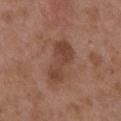Q: Was this lesion biopsied?
A: no biopsy performed (imaged during a skin exam)
Q: Patient demographics?
A: female, roughly 35 years of age
Q: Lesion size?
A: ≈6 mm
Q: How was this image acquired?
A: ~15 mm tile from a whole-body skin photo
Q: What did automated image analysis measure?
A: a footprint of about 11 mm² and a shape eccentricity near 0.9; a mean CIELAB color near L≈43 a*≈21 b*≈28, roughly 9 lightness units darker than nearby skin, and a normalized lesion–skin contrast near 7; a border-irregularity rating of about 4.5/10 and internal color variation of about 3 on a 0–10 scale
Q: What is the anatomic site?
A: the arm
Q: What lighting was used for the tile?
A: white-light illumination